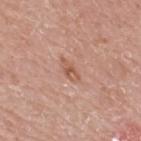  biopsy_status: not biopsied; imaged during a skin examination
  image:
    source: total-body photography crop
    field_of_view_mm: 15
  lighting: white-light
  site: upper back
  patient:
    sex: male
    age_approx: 75